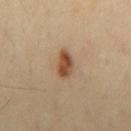No biopsy was performed on this lesion — it was imaged during a full skin examination and was not determined to be concerning.